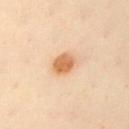Findings:
– workup — no biopsy performed (imaged during a skin exam)
– patient — female, about 60 years old
– image — total-body-photography crop, ~15 mm field of view
– size — about 3 mm
– lighting — cross-polarized
– anatomic site — the front of the torso